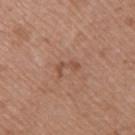Clinical impression:
The lesion was photographed on a routine skin check and not biopsied; there is no pathology result.
Context:
The lesion is on the right upper arm. A female patient in their 60s. Automated tile analysis of the lesion measured roughly 7 lightness units darker than nearby skin and a lesion-to-skin contrast of about 5.5 (normalized; higher = more distinct). And it measured a within-lesion color-variation index near 0/10 and radial color variation of about 0. Approximately 3 mm at its widest. Cropped from a total-body skin-imaging series; the visible field is about 15 mm. This is a white-light tile.